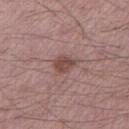notes: imaged on a skin check; not biopsied | anatomic site: the leg | image-analysis metrics: a normalized lesion–skin contrast near 8 | image: 15 mm crop, total-body photography | patient: male, approximately 50 years of age.